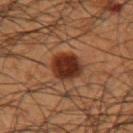Q: Was this lesion biopsied?
A: imaged on a skin check; not biopsied
Q: How large is the lesion?
A: ≈4 mm
Q: Lesion location?
A: the left forearm
Q: Who is the patient?
A: male, aged 48 to 52
Q: What kind of image is this?
A: total-body-photography crop, ~15 mm field of view